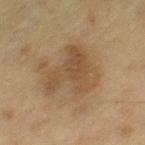Recorded during total-body skin imaging; not selected for excision or biopsy. The patient is a female approximately 55 years of age. This image is a 15 mm lesion crop taken from a total-body photograph. From the left thigh.